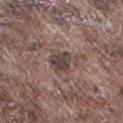Q: Was a biopsy performed?
A: total-body-photography surveillance lesion; no biopsy
Q: How large is the lesion?
A: ≈3 mm
Q: What lighting was used for the tile?
A: white-light illumination
Q: What is the imaging modality?
A: total-body-photography crop, ~15 mm field of view
Q: What are the patient's age and sex?
A: male, aged approximately 75
Q: Lesion location?
A: the leg